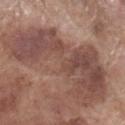Clinical impression: The lesion was tiled from a total-body skin photograph and was not biopsied. Image and clinical context: A male subject, aged 68 to 72. About 13 mm across. From the right lower leg. Imaged with white-light lighting. The total-body-photography lesion software estimated a footprint of about 55 mm², an eccentricity of roughly 0.9, and two-axis asymmetry of about 0.5. It also reported a lesion color around L≈47 a*≈20 b*≈23 in CIELAB, about 10 CIELAB-L* units darker than the surrounding skin, and a normalized border contrast of about 7.5. It also reported a border-irregularity index near 8/10 and a color-variation rating of about 5/10. The software also gave a classifier nevus-likeness of about 0/100 and lesion-presence confidence of about 75/100. A region of skin cropped from a whole-body photographic capture, roughly 15 mm wide.